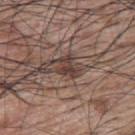Part of a total-body skin-imaging series; this lesion was reviewed on a skin check and was not flagged for biopsy.
Cropped from a total-body skin-imaging series; the visible field is about 15 mm.
A male subject approximately 70 years of age.
Located on the back.
Measured at roughly 2.5 mm in maximum diameter.